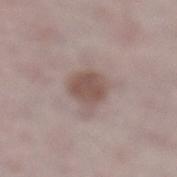Recorded during total-body skin imaging; not selected for excision or biopsy. A female patient, aged approximately 50. Automated image analysis of the tile measured a symmetry-axis asymmetry near 0.2. It also reported a lesion color around L≈51 a*≈16 b*≈21 in CIELAB and a normalized border contrast of about 8. It also reported a classifier nevus-likeness of about 60/100 and a lesion-detection confidence of about 100/100. A region of skin cropped from a whole-body photographic capture, roughly 15 mm wide. Located on the leg. Longest diameter approximately 3.5 mm. This is a white-light tile.